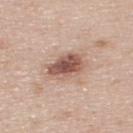Clinical summary: The lesion-visualizer software estimated an area of roughly 9 mm², an outline eccentricity of about 0.75 (0 = round, 1 = elongated), and a shape-asymmetry score of about 0.25 (0 = symmetric). It also reported a mean CIELAB color near L≈54 a*≈21 b*≈25 and roughly 15 lightness units darker than nearby skin. The analysis additionally found a classifier nevus-likeness of about 85/100 and a lesion-detection confidence of about 100/100. On the back. The patient is a male aged 43 to 47. Approximately 4 mm at its widest. The tile uses white-light illumination. Cropped from a whole-body photographic skin survey; the tile spans about 15 mm.